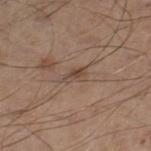The lesion was photographed on a routine skin check and not biopsied; there is no pathology result. An algorithmic analysis of the crop reported a lesion area of about 3.5 mm² and an outline eccentricity of about 0.85 (0 = round, 1 = elongated). And it measured a lesion color around L≈46 a*≈16 b*≈26 in CIELAB and a lesion-to-skin contrast of about 6.5 (normalized; higher = more distinct). The software also gave a color-variation rating of about 2.5/10. A male patient aged around 60. On the right thigh. A close-up tile cropped from a whole-body skin photograph, about 15 mm across. Captured under white-light illumination.